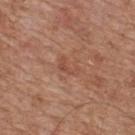Recorded during total-body skin imaging; not selected for excision or biopsy. The patient is a male aged 63–67. A 15 mm close-up tile from a total-body photography series done for melanoma screening. The lesion is on the back.